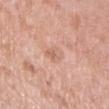The lesion's longest dimension is about 2.5 mm.
The lesion is located on the left upper arm.
A 15 mm close-up tile from a total-body photography series done for melanoma screening.
Automated tile analysis of the lesion measured a border-irregularity rating of about 2.5/10, a within-lesion color-variation index near 3/10, and peripheral color asymmetry of about 1. And it measured an automated nevus-likeness rating near 0 out of 100 and lesion-presence confidence of about 100/100.
Captured under white-light illumination.
The patient is a male roughly 50 years of age.